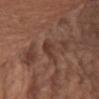Assessment: No biopsy was performed on this lesion — it was imaged during a full skin examination and was not determined to be concerning. Context: A 15 mm close-up extracted from a 3D total-body photography capture. From the left upper arm. A female patient, aged approximately 55.